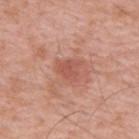Captured during whole-body skin photography for melanoma surveillance; the lesion was not biopsied.